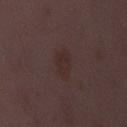Recorded during total-body skin imaging; not selected for excision or biopsy. Longest diameter approximately 4 mm. A close-up tile cropped from a whole-body skin photograph, about 15 mm across. On the lower back. Imaged with white-light lighting. A female patient in their mid- to late 30s.